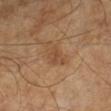Captured during whole-body skin photography for melanoma surveillance; the lesion was not biopsied.
The patient is a male aged around 65.
Cropped from a whole-body photographic skin survey; the tile spans about 15 mm.